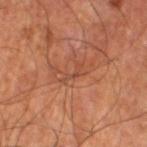| field | value |
|---|---|
| image source | 15 mm crop, total-body photography |
| anatomic site | the leg |
| patient | male, aged around 60 |
| lesion diameter | about 2.5 mm |